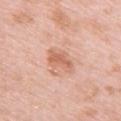The lesion was tiled from a total-body skin photograph and was not biopsied. A female subject, aged approximately 65. Imaged with white-light lighting. A region of skin cropped from a whole-body photographic capture, roughly 15 mm wide. The lesion is on the upper back. An algorithmic analysis of the crop reported internal color variation of about 2 on a 0–10 scale and peripheral color asymmetry of about 0.5.